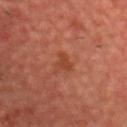workup: no biopsy performed (imaged during a skin exam) | acquisition: total-body-photography crop, ~15 mm field of view | patient: male, aged around 60 | site: the head or neck | tile lighting: cross-polarized illumination | automated lesion analysis: an eccentricity of roughly 0.6 and a shape-asymmetry score of about 0.45 (0 = symmetric); a border-irregularity index near 4/10 and radial color variation of about 0.5.